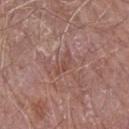Impression: No biopsy was performed on this lesion — it was imaged during a full skin examination and was not determined to be concerning. Image and clinical context: From the arm. Measured at roughly 3 mm in maximum diameter. A male subject aged around 70. Cropped from a whole-body photographic skin survey; the tile spans about 15 mm. The lesion-visualizer software estimated a footprint of about 3.5 mm², an eccentricity of roughly 0.85, and two-axis asymmetry of about 0.45. And it measured a lesion color around L≈48 a*≈22 b*≈24 in CIELAB and a lesion-to-skin contrast of about 5 (normalized; higher = more distinct).